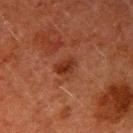| feature | finding |
|---|---|
| follow-up | no biopsy performed (imaged during a skin exam) |
| lesion size | ~2.5 mm (longest diameter) |
| acquisition | ~15 mm crop, total-body skin-cancer survey |
| lighting | cross-polarized |
| subject | male, approximately 60 years of age |
| site | the right upper arm |